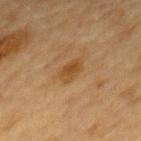biopsy status — catalogued during a skin exam; not biopsied | patient — female, roughly 60 years of age | tile lighting — cross-polarized | imaging modality — 15 mm crop, total-body photography | automated metrics — a detector confidence of about 100 out of 100 that the crop contains a lesion | site — the back | diameter — ≈3 mm.